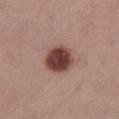notes: total-body-photography surveillance lesion; no biopsy
illumination: white-light illumination
location: the left thigh
patient: female, aged around 55
image: ~15 mm tile from a whole-body skin photo
size: ≈4 mm